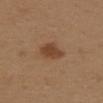Acquisition and patient details:
A female subject, aged around 40. Cropped from a whole-body photographic skin survey; the tile spans about 15 mm. The lesion is located on the upper back. Imaged with white-light lighting.